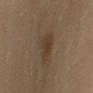follow-up=imaged on a skin check; not biopsied
subject=male, about 55 years old
imaging modality=~15 mm tile from a whole-body skin photo
diameter=about 3 mm
tile lighting=cross-polarized illumination
site=the right upper arm